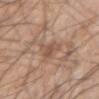Imaged during a routine full-body skin examination; the lesion was not biopsied and no histopathology is available. The lesion's longest dimension is about 5.5 mm. Imaged with white-light lighting. The lesion is on the arm. Cropped from a whole-body photographic skin survey; the tile spans about 15 mm. Automated tile analysis of the lesion measured a lesion area of about 6.5 mm², a shape eccentricity near 0.9, and a shape-asymmetry score of about 0.5 (0 = symmetric). The analysis additionally found a border-irregularity rating of about 6/10, a within-lesion color-variation index near 2.5/10, and radial color variation of about 1. A male subject, aged 63 to 67.